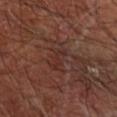Notes:
- workup — catalogued during a skin exam; not biopsied
- patient — male, approximately 65 years of age
- location — the right forearm
- acquisition — ~15 mm crop, total-body skin-cancer survey